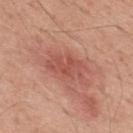This lesion was catalogued during total-body skin photography and was not selected for biopsy. Longest diameter approximately 5 mm. The lesion-visualizer software estimated a mean CIELAB color near L≈53 a*≈27 b*≈28, about 9 CIELAB-L* units darker than the surrounding skin, and a normalized lesion–skin contrast near 6. The analysis additionally found a nevus-likeness score of about 5/100 and a detector confidence of about 100 out of 100 that the crop contains a lesion. A 15 mm close-up tile from a total-body photography series done for melanoma screening. Captured under white-light illumination. A male subject aged around 55. The lesion is on the upper back.